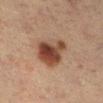A female patient, approximately 55 years of age. On the right lower leg. A region of skin cropped from a whole-body photographic capture, roughly 15 mm wide. Imaged with cross-polarized lighting. An algorithmic analysis of the crop reported a classifier nevus-likeness of about 95/100 and a detector confidence of about 100 out of 100 that the crop contains a lesion. Longest diameter approximately 5 mm.